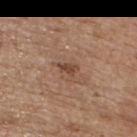Q: Was this lesion biopsied?
A: catalogued during a skin exam; not biopsied
Q: Where on the body is the lesion?
A: the right upper arm
Q: Lesion size?
A: ≈2.5 mm
Q: What are the patient's age and sex?
A: female, aged 63–67
Q: What kind of image is this?
A: total-body-photography crop, ~15 mm field of view
Q: What lighting was used for the tile?
A: white-light illumination
Q: Automated lesion metrics?
A: a lesion area of about 3 mm², a shape eccentricity near 0.8, and two-axis asymmetry of about 0.35; a mean CIELAB color near L≈45 a*≈19 b*≈28 and a normalized lesion–skin contrast near 7